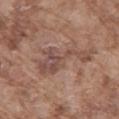Case summary:
• notes: total-body-photography surveillance lesion; no biopsy
• subject: male, aged 73 to 77
• body site: the mid back
• lesion diameter: about 7 mm
• image: ~15 mm crop, total-body skin-cancer survey
• lighting: white-light
• automated lesion analysis: a footprint of about 13 mm², a shape eccentricity near 0.9, and a shape-asymmetry score of about 0.7 (0 = symmetric); a mean CIELAB color near L≈48 a*≈19 b*≈24 and a normalized lesion–skin contrast near 6.5; an automated nevus-likeness rating near 0 out of 100 and lesion-presence confidence of about 95/100